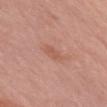The lesion was tiled from a total-body skin photograph and was not biopsied. Approximately 2.5 mm at its widest. The lesion is on the right upper arm. A female subject aged approximately 60. Captured under white-light illumination. Cropped from a whole-body photographic skin survey; the tile spans about 15 mm.